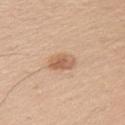Imaged during a routine full-body skin examination; the lesion was not biopsied and no histopathology is available. From the arm. Cropped from a whole-body photographic skin survey; the tile spans about 15 mm. Imaged with white-light lighting. A male subject, approximately 50 years of age. The recorded lesion diameter is about 3.5 mm.